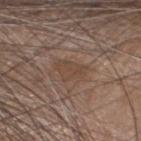<lesion>
<biopsy_status>not biopsied; imaged during a skin examination</biopsy_status>
<lighting>white-light</lighting>
<site>head or neck</site>
<image>
  <source>total-body photography crop</source>
  <field_of_view_mm>15</field_of_view_mm>
</image>
<patient>
  <sex>male</sex>
  <age_approx>55</age_approx>
</patient>
<lesion_size>
  <long_diameter_mm_approx>3.5</long_diameter_mm_approx>
</lesion_size>
</lesion>